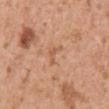Clinical impression: The lesion was photographed on a routine skin check and not biopsied; there is no pathology result. Acquisition and patient details: An algorithmic analysis of the crop reported a lesion–skin lightness drop of about 7 and a lesion-to-skin contrast of about 5 (normalized; higher = more distinct). Captured under white-light illumination. Longest diameter approximately 3 mm. From the right upper arm. A female subject aged around 40. Cropped from a total-body skin-imaging series; the visible field is about 15 mm.